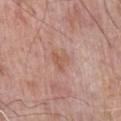biopsy status — no biopsy performed (imaged during a skin exam)
lesion diameter — ≈3 mm
image — ~15 mm tile from a whole-body skin photo
subject — male, about 70 years old
lighting — white-light illumination
body site — the chest
automated metrics — a mean CIELAB color near L≈57 a*≈21 b*≈29, roughly 7 lightness units darker than nearby skin, and a lesion-to-skin contrast of about 5.5 (normalized; higher = more distinct)